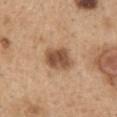Clinical impression:
No biopsy was performed on this lesion — it was imaged during a full skin examination and was not determined to be concerning.
Background:
The total-body-photography lesion software estimated border irregularity of about 1.5 on a 0–10 scale, a color-variation rating of about 4.5/10, and a peripheral color-asymmetry measure near 1.5. It also reported an automated nevus-likeness rating near 65 out of 100 and lesion-presence confidence of about 100/100. The patient is a female approximately 60 years of age. Located on the upper back. The tile uses white-light illumination. Approximately 4 mm at its widest. A close-up tile cropped from a whole-body skin photograph, about 15 mm across.